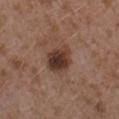Q: Was a biopsy performed?
A: imaged on a skin check; not biopsied
Q: How large is the lesion?
A: ~4 mm (longest diameter)
Q: What is the imaging modality?
A: ~15 mm crop, total-body skin-cancer survey
Q: What is the anatomic site?
A: the left forearm
Q: What are the patient's age and sex?
A: female, aged around 35
Q: Illumination type?
A: white-light